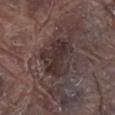Q: Was this lesion biopsied?
A: catalogued during a skin exam; not biopsied
Q: Automated lesion metrics?
A: lesion-presence confidence of about 95/100
Q: What is the anatomic site?
A: the leg
Q: Who is the patient?
A: male, aged 78–82
Q: Lesion size?
A: ~5.5 mm (longest diameter)
Q: What kind of image is this?
A: ~15 mm tile from a whole-body skin photo